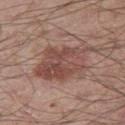Case summary:
• workup: catalogued during a skin exam; not biopsied
• anatomic site: the leg
• patient: male, about 50 years old
• imaging modality: ~15 mm tile from a whole-body skin photo
• tile lighting: white-light illumination
• size: about 7 mm